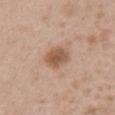Recorded during total-body skin imaging; not selected for excision or biopsy. A 15 mm close-up tile from a total-body photography series done for melanoma screening. Approximately 3 mm at its widest. The lesion is located on the abdomen. A male subject aged 63 to 67. Captured under white-light illumination.